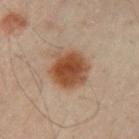Impression: Imaged during a routine full-body skin examination; the lesion was not biopsied and no histopathology is available. Context: Automated image analysis of the tile measured a lesion area of about 15 mm² and a shape-asymmetry score of about 0.15 (0 = symmetric). It also reported about 12 CIELAB-L* units darker than the surrounding skin. The lesion is located on the left upper arm. About 5 mm across. A region of skin cropped from a whole-body photographic capture, roughly 15 mm wide. A male subject, aged approximately 50. This is a cross-polarized tile.